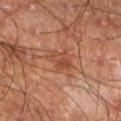Image and clinical context:
This image is a 15 mm lesion crop taken from a total-body photograph. A male patient, about 60 years old. An algorithmic analysis of the crop reported a shape-asymmetry score of about 0.4 (0 = symmetric). The software also gave a nevus-likeness score of about 0/100. This is a cross-polarized tile. The lesion is on the left lower leg.